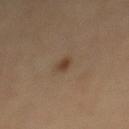  biopsy_status: not biopsied; imaged during a skin examination
  automated_metrics:
    border_irregularity_0_10: 3.0
    color_variation_0_10: 0.5
    peripheral_color_asymmetry: 0.0
  patient:
    sex: male
    age_approx: 60
  image:
    source: total-body photography crop
    field_of_view_mm: 15
  site: mid back
  lighting: cross-polarized
  lesion_size:
    long_diameter_mm_approx: 2.0